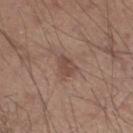Part of a total-body skin-imaging series; this lesion was reviewed on a skin check and was not flagged for biopsy. From the left lower leg. The subject is a male in their 30s. Cropped from a total-body skin-imaging series; the visible field is about 15 mm.